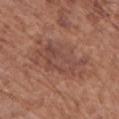<lesion>
  <biopsy_status>not biopsied; imaged during a skin examination</biopsy_status>
  <lesion_size>
    <long_diameter_mm_approx>7.0</long_diameter_mm_approx>
  </lesion_size>
  <patient>
    <sex>female</sex>
    <age_approx>75</age_approx>
  </patient>
  <image>
    <source>total-body photography crop</source>
    <field_of_view_mm>15</field_of_view_mm>
  </image>
  <site>upper back</site>
  <lighting>white-light</lighting>
</lesion>